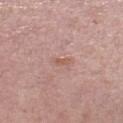This lesion was catalogued during total-body skin photography and was not selected for biopsy. Located on the right lower leg. A 15 mm close-up extracted from a 3D total-body photography capture. A female subject roughly 50 years of age.